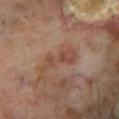The lesion was tiled from a total-body skin photograph and was not biopsied.
Measured at roughly 5 mm in maximum diameter.
Imaged with cross-polarized lighting.
A lesion tile, about 15 mm wide, cut from a 3D total-body photograph.
A male subject, about 70 years old.
Located on the leg.